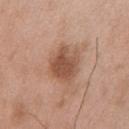- workup — total-body-photography surveillance lesion; no biopsy
- patient — male, aged around 50
- image source — ~15 mm tile from a whole-body skin photo
- body site — the chest